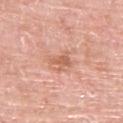workup = no biopsy performed (imaged during a skin exam)
image source = ~15 mm tile from a whole-body skin photo
body site = the left upper arm
patient = male, aged 78–82